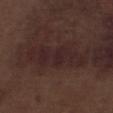Imaged during a routine full-body skin examination; the lesion was not biopsied and no histopathology is available. Located on the left lower leg. Measured at roughly 7.5 mm in maximum diameter. Automated image analysis of the tile measured a color-variation rating of about 3/10 and a peripheral color-asymmetry measure near 1. It also reported lesion-presence confidence of about 60/100. Imaged with white-light lighting. A male subject, aged 68–72. A lesion tile, about 15 mm wide, cut from a 3D total-body photograph.